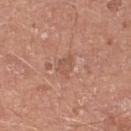Q: Was a biopsy performed?
A: total-body-photography surveillance lesion; no biopsy
Q: Who is the patient?
A: male, about 80 years old
Q: What did automated image analysis measure?
A: an eccentricity of roughly 0.65
Q: What kind of image is this?
A: 15 mm crop, total-body photography
Q: Lesion size?
A: about 2.5 mm
Q: What is the anatomic site?
A: the leg
Q: Illumination type?
A: white-light illumination